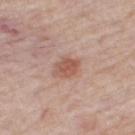Q: Is there a histopathology result?
A: imaged on a skin check; not biopsied
Q: What did automated image analysis measure?
A: a footprint of about 5 mm² and a shape-asymmetry score of about 0.2 (0 = symmetric); a mean CIELAB color near L≈55 a*≈22 b*≈27, about 10 CIELAB-L* units darker than the surrounding skin, and a lesion-to-skin contrast of about 7 (normalized; higher = more distinct); a classifier nevus-likeness of about 90/100
Q: What kind of image is this?
A: ~15 mm crop, total-body skin-cancer survey
Q: What are the patient's age and sex?
A: female, aged around 60
Q: What is the anatomic site?
A: the right thigh
Q: Illumination type?
A: white-light illumination